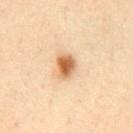| field | value |
|---|---|
| biopsy status | no biopsy performed (imaged during a skin exam) |